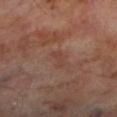<record>
  <biopsy_status>not biopsied; imaged during a skin examination</biopsy_status>
  <patient>
    <sex>male</sex>
    <age_approx>70</age_approx>
  </patient>
  <lesion_size>
    <long_diameter_mm_approx>3.0</long_diameter_mm_approx>
  </lesion_size>
  <automated_metrics>
    <border_irregularity_0_10>4.0</border_irregularity_0_10>
    <peripheral_color_asymmetry>0.0</peripheral_color_asymmetry>
  </automated_metrics>
  <lighting>cross-polarized</lighting>
  <site>leg</site>
  <image>
    <source>total-body photography crop</source>
    <field_of_view_mm>15</field_of_view_mm>
  </image>
</record>